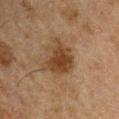{"biopsy_status": "not biopsied; imaged during a skin examination", "automated_metrics": {"area_mm2_approx": 13.0, "eccentricity": 0.55, "shape_asymmetry": 0.35, "cielab_L": 32, "cielab_a": 15, "cielab_b": 27, "vs_skin_darker_L": 9.0, "vs_skin_contrast_norm": 8.5, "color_variation_0_10": 3.0, "peripheral_color_asymmetry": 1.0, "nevus_likeness_0_100": 60, "lesion_detection_confidence_0_100": 100}, "lesion_size": {"long_diameter_mm_approx": 4.5}, "site": "left upper arm", "image": {"source": "total-body photography crop", "field_of_view_mm": 15}, "patient": {"sex": "male", "age_approx": 50}}